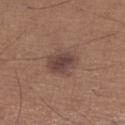{"biopsy_status": "not biopsied; imaged during a skin examination", "lighting": "white-light", "automated_metrics": {"border_irregularity_0_10": 2.0, "color_variation_0_10": 3.5, "peripheral_color_asymmetry": 1.0, "nevus_likeness_0_100": 70, "lesion_detection_confidence_0_100": 100}, "patient": {"sex": "male", "age_approx": 65}, "lesion_size": {"long_diameter_mm_approx": 3.5}, "site": "right lower leg", "image": {"source": "total-body photography crop", "field_of_view_mm": 15}}